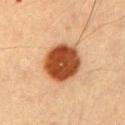This lesion was catalogued during total-body skin photography and was not selected for biopsy. Cropped from a total-body skin-imaging series; the visible field is about 15 mm. A male subject aged 53 to 57. Located on the right upper arm. The lesion's longest dimension is about 5 mm.